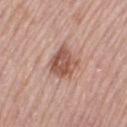Q: Was this lesion biopsied?
A: catalogued during a skin exam; not biopsied
Q: What is the imaging modality?
A: 15 mm crop, total-body photography
Q: Who is the patient?
A: female, aged 63–67
Q: What lighting was used for the tile?
A: white-light illumination
Q: Lesion location?
A: the left thigh